A close-up tile cropped from a whole-body skin photograph, about 15 mm across; the lesion is located on the upper back; a female patient, aged around 65: 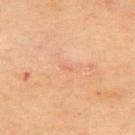On biopsy, histopathology showed a superficial basal cell carcinoma.